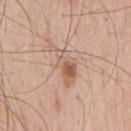On the chest.
The tile uses white-light illumination.
Longest diameter approximately 6 mm.
A lesion tile, about 15 mm wide, cut from a 3D total-body photograph.
A male subject aged around 60.
An algorithmic analysis of the crop reported roughly 10 lightness units darker than nearby skin. And it measured border irregularity of about 7 on a 0–10 scale, internal color variation of about 6 on a 0–10 scale, and peripheral color asymmetry of about 1.5.